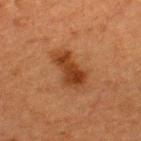Q: Was this lesion biopsied?
A: catalogued during a skin exam; not biopsied
Q: Automated lesion metrics?
A: a border-irregularity index near 3.5/10, internal color variation of about 3.5 on a 0–10 scale, and radial color variation of about 1; a lesion-detection confidence of about 100/100
Q: Lesion size?
A: ~4.5 mm (longest diameter)
Q: Lesion location?
A: the upper back
Q: Patient demographics?
A: male, approximately 50 years of age
Q: What is the imaging modality?
A: 15 mm crop, total-body photography
Q: Illumination type?
A: cross-polarized illumination